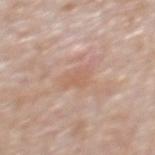Impression: The lesion was photographed on a routine skin check and not biopsied; there is no pathology result. Acquisition and patient details: A male subject, about 75 years old. Located on the mid back. Imaged with white-light lighting. A 15 mm crop from a total-body photograph taken for skin-cancer surveillance. An algorithmic analysis of the crop reported a mean CIELAB color near L≈61 a*≈19 b*≈29, roughly 6 lightness units darker than nearby skin, and a normalized lesion–skin contrast near 4.5. And it measured a border-irregularity index near 4/10 and internal color variation of about 2 on a 0–10 scale. The analysis additionally found a classifier nevus-likeness of about 0/100 and a lesion-detection confidence of about 100/100. About 3.5 mm across.